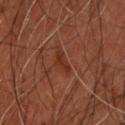{"patient": {"sex": "male", "age_approx": 45}, "lighting": "cross-polarized", "site": "back", "image": {"source": "total-body photography crop", "field_of_view_mm": 15}, "automated_metrics": {"eccentricity": 0.85, "shape_asymmetry": 0.5, "cielab_L": 30, "cielab_a": 25, "cielab_b": 28, "vs_skin_darker_L": 6.0, "vs_skin_contrast_norm": 6.5}}